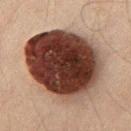subject = male, about 45 years old | lighting = cross-polarized | lesion diameter = about 10 mm | image = 15 mm crop, total-body photography | location = the chest.